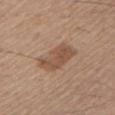  biopsy_status: not biopsied; imaged during a skin examination
  patient:
    sex: male
    age_approx: 60
  automated_metrics:
    border_irregularity_0_10: 3.0
    color_variation_0_10: 2.5
    peripheral_color_asymmetry: 1.0
    nevus_likeness_0_100: 25
    lesion_detection_confidence_0_100: 100
  site: left upper arm
  lesion_size:
    long_diameter_mm_approx: 5.0
  image:
    source: total-body photography crop
    field_of_view_mm: 15